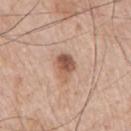Q: Was this lesion biopsied?
A: total-body-photography surveillance lesion; no biopsy
Q: Lesion size?
A: about 3.5 mm
Q: Patient demographics?
A: male, about 65 years old
Q: How was the tile lit?
A: white-light
Q: Where on the body is the lesion?
A: the right upper arm
Q: How was this image acquired?
A: ~15 mm crop, total-body skin-cancer survey